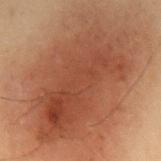Captured during whole-body skin photography for melanoma surveillance; the lesion was not biopsied.
This is a cross-polarized tile.
The recorded lesion diameter is about 14 mm.
The lesion is located on the mid back.
The patient is a male aged approximately 55.
Cropped from a whole-body photographic skin survey; the tile spans about 15 mm.